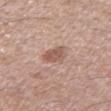workup: no biopsy performed (imaged during a skin exam)
subject: male, approximately 65 years of age
size: about 3 mm
image source: 15 mm crop, total-body photography
body site: the leg